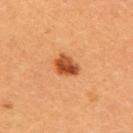Captured during whole-body skin photography for melanoma surveillance; the lesion was not biopsied.